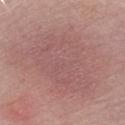{
  "site": "left upper arm",
  "patient": {
    "sex": "male",
    "age_approx": 65
  },
  "image": {
    "source": "total-body photography crop",
    "field_of_view_mm": 15
  },
  "lesion_size": {
    "long_diameter_mm_approx": 12.0
  },
  "lighting": "white-light"
}